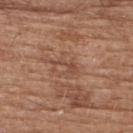The lesion was photographed on a routine skin check and not biopsied; there is no pathology result. The total-body-photography lesion software estimated an area of roughly 3.5 mm². And it measured a lesion color around L≈48 a*≈21 b*≈30 in CIELAB and roughly 7 lightness units darker than nearby skin. The software also gave a border-irregularity rating of about 7/10, a color-variation rating of about 1/10, and a peripheral color-asymmetry measure near 0. Measured at roughly 3.5 mm in maximum diameter. A male subject, aged around 75. The lesion is located on the upper back. A lesion tile, about 15 mm wide, cut from a 3D total-body photograph.